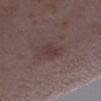Case summary:
• workup — catalogued during a skin exam; not biopsied
• anatomic site — the right lower leg
• automated metrics — a lesion area of about 4 mm², a shape eccentricity near 0.8, and a shape-asymmetry score of about 0.25 (0 = symmetric); a lesion–skin lightness drop of about 6 and a normalized lesion–skin contrast near 5.5; a within-lesion color-variation index near 1/10 and a peripheral color-asymmetry measure near 0.5; a nevus-likeness score of about 0/100 and a detector confidence of about 100 out of 100 that the crop contains a lesion
• imaging modality — 15 mm crop, total-body photography
• lesion size — about 2.5 mm
• lighting — white-light illumination
• patient — female, in their mid- to late 30s